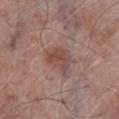Case summary:
• workup · no biopsy performed (imaged during a skin exam)
• image · ~15 mm tile from a whole-body skin photo
• subject · male, approximately 70 years of age
• site · the leg
• tile lighting · white-light